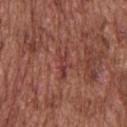Clinical impression:
Captured during whole-body skin photography for melanoma surveillance; the lesion was not biopsied.
Acquisition and patient details:
On the upper back. This is a white-light tile. A 15 mm crop from a total-body photograph taken for skin-cancer surveillance. A male subject aged approximately 75. Measured at roughly 3.5 mm in maximum diameter.